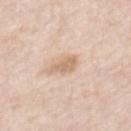Recorded during total-body skin imaging; not selected for excision or biopsy. A 15 mm close-up tile from a total-body photography series done for melanoma screening. This is a white-light tile. Longest diameter approximately 3.5 mm. A male patient aged approximately 60. The lesion is on the mid back.